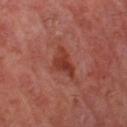biopsy status: catalogued during a skin exam; not biopsied
automated metrics: a lesion-to-skin contrast of about 8.5 (normalized; higher = more distinct); a border-irregularity rating of about 5/10, a within-lesion color-variation index near 3/10, and peripheral color asymmetry of about 1
image source: ~15 mm crop, total-body skin-cancer survey
patient: male, aged 68 to 72
lighting: cross-polarized illumination
site: the left thigh